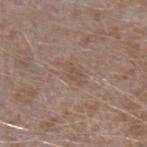Clinical impression: Imaged during a routine full-body skin examination; the lesion was not biopsied and no histopathology is available. Context: A region of skin cropped from a whole-body photographic capture, roughly 15 mm wide. On the right forearm. Captured under white-light illumination. A male patient, aged approximately 45. Measured at roughly 2.5 mm in maximum diameter. Automated tile analysis of the lesion measured a lesion area of about 2.5 mm², a shape eccentricity near 0.85, and a shape-asymmetry score of about 0.2 (0 = symmetric). It also reported roughly 5 lightness units darker than nearby skin and a normalized border contrast of about 4.5. It also reported a within-lesion color-variation index near 0.5/10 and a peripheral color-asymmetry measure near 0. The software also gave a classifier nevus-likeness of about 0/100 and a detector confidence of about 100 out of 100 that the crop contains a lesion.